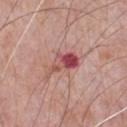Captured during whole-body skin photography for melanoma surveillance; the lesion was not biopsied.
A male patient aged approximately 70.
About 5 mm across.
A roughly 15 mm field-of-view crop from a total-body skin photograph.
The lesion is on the chest.